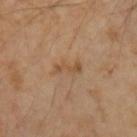The lesion was tiled from a total-body skin photograph and was not biopsied. The tile uses cross-polarized illumination. Cropped from a total-body skin-imaging series; the visible field is about 15 mm. The lesion's longest dimension is about 3 mm. An algorithmic analysis of the crop reported two-axis asymmetry of about 0.4. It also reported roughly 7 lightness units darker than nearby skin and a normalized border contrast of about 6. It also reported border irregularity of about 5.5 on a 0–10 scale, a within-lesion color-variation index near 0/10, and a peripheral color-asymmetry measure near 0. The software also gave an automated nevus-likeness rating near 0 out of 100 and a lesion-detection confidence of about 100/100. Located on the left upper arm. A female subject in their mid-40s.